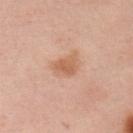follow-up: no biopsy performed (imaged during a skin exam)
patient: female, aged approximately 55
diameter: ≈3 mm
anatomic site: the chest
automated lesion analysis: a footprint of about 6 mm², an outline eccentricity of about 0.7 (0 = round, 1 = elongated), and a symmetry-axis asymmetry near 0.25; a lesion color around L≈49 a*≈18 b*≈28 in CIELAB, about 7 CIELAB-L* units darker than the surrounding skin, and a normalized border contrast of about 6.5; a border-irregularity index near 2/10, a color-variation rating of about 2.5/10, and a peripheral color-asymmetry measure near 1
acquisition: ~15 mm tile from a whole-body skin photo